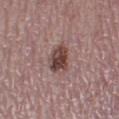| feature | finding |
|---|---|
| notes | imaged on a skin check; not biopsied |
| patient | female, roughly 50 years of age |
| image | ~15 mm crop, total-body skin-cancer survey |
| location | the left thigh |